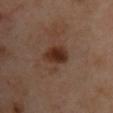automated lesion analysis: an automated nevus-likeness rating near 95 out of 100 and a detector confidence of about 100 out of 100 that the crop contains a lesion | imaging modality: ~15 mm crop, total-body skin-cancer survey | patient: female, approximately 40 years of age | lesion size: about 3.5 mm | body site: the chest.